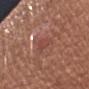Findings:
– notes — total-body-photography surveillance lesion; no biopsy
– site — the left upper arm
– diameter — ~3 mm (longest diameter)
– image — 15 mm crop, total-body photography
– patient — female, in their mid- to late 60s